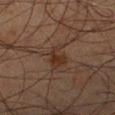Case summary:
• workup: imaged on a skin check; not biopsied
• subject: male, about 70 years old
• acquisition: 15 mm crop, total-body photography
• site: the right lower leg